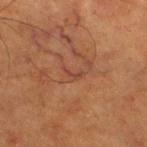biopsy status: imaged on a skin check; not biopsied
image source: 15 mm crop, total-body photography
lighting: cross-polarized
body site: the right lower leg
image-analysis metrics: an area of roughly 2.5 mm², an outline eccentricity of about 0.85 (0 = round, 1 = elongated), and a shape-asymmetry score of about 0.65 (0 = symmetric); a mean CIELAB color near L≈35 a*≈20 b*≈25, roughly 5 lightness units darker than nearby skin, and a lesion-to-skin contrast of about 5 (normalized; higher = more distinct); border irregularity of about 6.5 on a 0–10 scale and a peripheral color-asymmetry measure near 0; a nevus-likeness score of about 0/100 and lesion-presence confidence of about 70/100
subject: male, in their mid- to late 60s
diameter: ≈2.5 mm